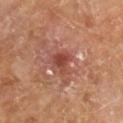notes = imaged on a skin check; not biopsied | image = 15 mm crop, total-body photography | automated lesion analysis = a mean CIELAB color near L≈44 a*≈27 b*≈28, a lesion–skin lightness drop of about 10, and a normalized lesion–skin contrast near 7.5; border irregularity of about 4 on a 0–10 scale, a within-lesion color-variation index near 4.5/10, and peripheral color asymmetry of about 1.5 | patient = male, in their mid- to late 60s | lesion diameter = ~3 mm (longest diameter).